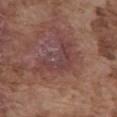Q: Was a biopsy performed?
A: imaged on a skin check; not biopsied
Q: What are the patient's age and sex?
A: male, roughly 75 years of age
Q: What is the anatomic site?
A: the front of the torso
Q: How large is the lesion?
A: ~5.5 mm (longest diameter)
Q: What is the imaging modality?
A: ~15 mm crop, total-body skin-cancer survey